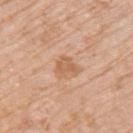Q: Is there a histopathology result?
A: no biopsy performed (imaged during a skin exam)
Q: Who is the patient?
A: female, aged approximately 75
Q: Lesion size?
A: ~3 mm (longest diameter)
Q: What is the anatomic site?
A: the left upper arm
Q: What lighting was used for the tile?
A: white-light
Q: What kind of image is this?
A: 15 mm crop, total-body photography